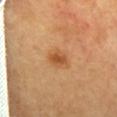Case summary:
– workup · imaged on a skin check; not biopsied
– imaging modality · ~15 mm tile from a whole-body skin photo
– automated metrics · an area of roughly 4.5 mm², a shape eccentricity near 0.7, and two-axis asymmetry of about 0.2; about 10 CIELAB-L* units darker than the surrounding skin and a lesion-to-skin contrast of about 7.5 (normalized; higher = more distinct); a border-irregularity index near 2/10, internal color variation of about 2.5 on a 0–10 scale, and radial color variation of about 0.5
– subject · female, approximately 60 years of age
– anatomic site · the mid back